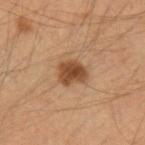workup: total-body-photography surveillance lesion; no biopsy
imaging modality: total-body-photography crop, ~15 mm field of view
lighting: cross-polarized illumination
subject: male, aged approximately 35
TBP lesion metrics: a lesion color around L≈46 a*≈21 b*≈34 in CIELAB and a lesion–skin lightness drop of about 13; a border-irregularity index near 2.5/10, a within-lesion color-variation index near 3/10, and a peripheral color-asymmetry measure near 1; a nevus-likeness score of about 95/100
body site: the left upper arm